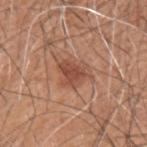Impression:
Part of a total-body skin-imaging series; this lesion was reviewed on a skin check and was not flagged for biopsy.
Clinical summary:
On the upper back. The subject is a male aged 58 to 62. A 15 mm crop from a total-body photograph taken for skin-cancer surveillance.